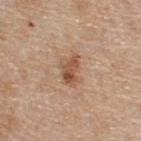Q: Was this lesion biopsied?
A: catalogued during a skin exam; not biopsied
Q: Who is the patient?
A: male, aged approximately 55
Q: Lesion size?
A: about 3.5 mm
Q: Where on the body is the lesion?
A: the upper back
Q: How was this image acquired?
A: ~15 mm tile from a whole-body skin photo
Q: What lighting was used for the tile?
A: white-light illumination